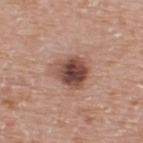This is a white-light tile. A male subject, about 75 years old. A 15 mm close-up tile from a total-body photography series done for melanoma screening. An algorithmic analysis of the crop reported an area of roughly 12 mm², a shape eccentricity near 0.5, and two-axis asymmetry of about 0.2. The software also gave an average lesion color of about L≈48 a*≈21 b*≈25 (CIELAB) and a normalized lesion–skin contrast near 10.5. The analysis additionally found a border-irregularity rating of about 2/10 and radial color variation of about 2.5. From the back.